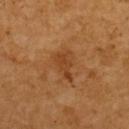{"biopsy_status": "not biopsied; imaged during a skin examination", "lighting": "cross-polarized", "image": {"source": "total-body photography crop", "field_of_view_mm": 15}, "lesion_size": {"long_diameter_mm_approx": 3.5}, "site": "upper back", "patient": {"sex": "female", "age_approx": 55}}